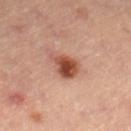Notes:
- site: the right lower leg
- lesion diameter: ≈3.5 mm
- subject: female, aged 43 to 47
- image source: total-body-photography crop, ~15 mm field of view